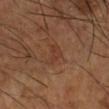follow-up — imaged on a skin check; not biopsied
image — 15 mm crop, total-body photography
patient — male, aged around 65
site — the leg